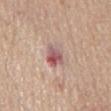Recorded during total-body skin imaging; not selected for excision or biopsy.
A male subject, roughly 65 years of age.
A 15 mm close-up tile from a total-body photography series done for melanoma screening.
Located on the front of the torso.